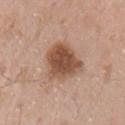Case summary:
- workup · no biopsy performed (imaged during a skin exam)
- tile lighting · white-light
- diameter · about 5 mm
- patient · male, about 55 years old
- TBP lesion metrics · an average lesion color of about L≈51 a*≈20 b*≈30 (CIELAB), about 14 CIELAB-L* units darker than the surrounding skin, and a normalized border contrast of about 10; internal color variation of about 4 on a 0–10 scale and a peripheral color-asymmetry measure near 1
- image source · ~15 mm crop, total-body skin-cancer survey
- anatomic site · the left upper arm